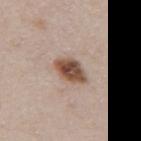Clinical impression: Part of a total-body skin-imaging series; this lesion was reviewed on a skin check and was not flagged for biopsy. Context: The patient is a female in their 40s. A 15 mm close-up extracted from a 3D total-body photography capture. The lesion is located on the back.